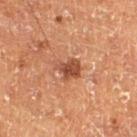This lesion was catalogued during total-body skin photography and was not selected for biopsy. The patient is a male in their mid- to late 70s. A close-up tile cropped from a whole-body skin photograph, about 15 mm across. From the leg.